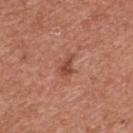Part of a total-body skin-imaging series; this lesion was reviewed on a skin check and was not flagged for biopsy.
Automated tile analysis of the lesion measured a mean CIELAB color near L≈48 a*≈27 b*≈29 and a lesion-to-skin contrast of about 7 (normalized; higher = more distinct). It also reported border irregularity of about 3.5 on a 0–10 scale, internal color variation of about 2 on a 0–10 scale, and peripheral color asymmetry of about 0.5. It also reported a classifier nevus-likeness of about 5/100 and a detector confidence of about 100 out of 100 that the crop contains a lesion.
From the chest.
A male patient, aged 43–47.
Cropped from a whole-body photographic skin survey; the tile spans about 15 mm.
Captured under white-light illumination.
Measured at roughly 3 mm in maximum diameter.